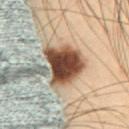Q: Who is the patient?
A: male, about 55 years old
Q: Illumination type?
A: cross-polarized
Q: Where on the body is the lesion?
A: the abdomen
Q: What is the lesion's diameter?
A: ~4.5 mm (longest diameter)
Q: What is the imaging modality?
A: 15 mm crop, total-body photography